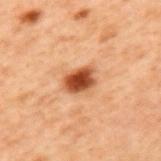Recorded during total-body skin imaging; not selected for excision or biopsy. Automated tile analysis of the lesion measured a footprint of about 6.5 mm², an outline eccentricity of about 0.7 (0 = round, 1 = elongated), and two-axis asymmetry of about 0.2. The analysis additionally found a border-irregularity index near 1.5/10, a color-variation rating of about 4.5/10, and a peripheral color-asymmetry measure near 1. A male subject aged around 70. On the mid back. Captured under cross-polarized illumination. Cropped from a total-body skin-imaging series; the visible field is about 15 mm. Approximately 3 mm at its widest.